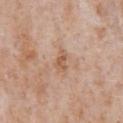Q: Is there a histopathology result?
A: total-body-photography surveillance lesion; no biopsy
Q: How was the tile lit?
A: white-light illumination
Q: What are the patient's age and sex?
A: male, roughly 65 years of age
Q: Where on the body is the lesion?
A: the chest
Q: Lesion size?
A: ≈3 mm
Q: What kind of image is this?
A: 15 mm crop, total-body photography